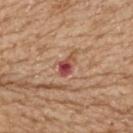Assessment: Part of a total-body skin-imaging series; this lesion was reviewed on a skin check and was not flagged for biopsy. Context: From the back. The tile uses white-light illumination. About 2.5 mm across. The patient is a female about 70 years old. Cropped from a total-body skin-imaging series; the visible field is about 15 mm.